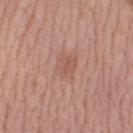{"patient": {"sex": "female", "age_approx": 40}, "lesion_size": {"long_diameter_mm_approx": 2.5}, "lighting": "white-light", "site": "left thigh", "image": {"source": "total-body photography crop", "field_of_view_mm": 15}, "automated_metrics": {"area_mm2_approx": 5.5, "eccentricity": 0.5, "vs_skin_darker_L": 6.0, "vs_skin_contrast_norm": 5.0, "nevus_likeness_0_100": 5}}